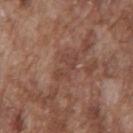notes = imaged on a skin check; not biopsied
size = ~4 mm (longest diameter)
image = total-body-photography crop, ~15 mm field of view
patient = male, about 75 years old
location = the chest